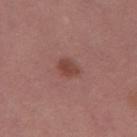Imaged during a routine full-body skin examination; the lesion was not biopsied and no histopathology is available. The tile uses white-light illumination. Approximately 2.5 mm at its widest. A lesion tile, about 15 mm wide, cut from a 3D total-body photograph. A female patient aged around 50. The lesion is located on the leg.